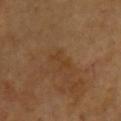Clinical impression: Part of a total-body skin-imaging series; this lesion was reviewed on a skin check and was not flagged for biopsy. Clinical summary: Automated image analysis of the tile measured a within-lesion color-variation index near 0/10 and peripheral color asymmetry of about 0. The software also gave a nevus-likeness score of about 0/100 and a lesion-detection confidence of about 100/100. The subject is a male aged 63 to 67. Located on the chest. The recorded lesion diameter is about 3 mm. A lesion tile, about 15 mm wide, cut from a 3D total-body photograph. Captured under cross-polarized illumination.